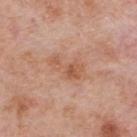Assessment:
This lesion was catalogued during total-body skin photography and was not selected for biopsy.
Background:
A male subject, approximately 75 years of age. A 15 mm close-up tile from a total-body photography series done for melanoma screening. On the upper back.